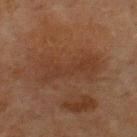Notes:
– biopsy status · no biopsy performed (imaged during a skin exam)
– subject · male, approximately 65 years of age
– acquisition · 15 mm crop, total-body photography
– illumination · cross-polarized illumination
– location · the front of the torso
– lesion diameter · about 7 mm